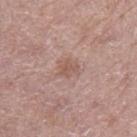Acquisition and patient details: A 15 mm close-up extracted from a 3D total-body photography capture. The lesion is located on the right thigh. A female subject aged approximately 75. Approximately 3 mm at its widest.